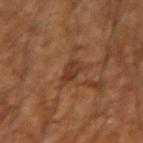About 2.5 mm across. A 15 mm close-up extracted from a 3D total-body photography capture. Imaged with cross-polarized lighting. The lesion-visualizer software estimated a shape eccentricity near 0.8 and two-axis asymmetry of about 0.25. From the arm. A male patient, aged approximately 55.